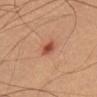{"biopsy_status": "not biopsied; imaged during a skin examination", "lighting": "cross-polarized", "site": "chest", "lesion_size": {"long_diameter_mm_approx": 2.0}, "patient": {"sex": "male", "age_approx": 45}, "image": {"source": "total-body photography crop", "field_of_view_mm": 15}}